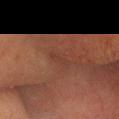{
  "biopsy_status": "not biopsied; imaged during a skin examination",
  "site": "head or neck",
  "patient": {
    "sex": "male",
    "age_approx": 60
  },
  "image": {
    "source": "total-body photography crop",
    "field_of_view_mm": 15
  }
}